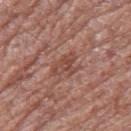Assessment: Part of a total-body skin-imaging series; this lesion was reviewed on a skin check and was not flagged for biopsy. Acquisition and patient details: A male patient, in their 80s. The lesion-visualizer software estimated a lesion area of about 5.5 mm² and an eccentricity of roughly 0.75. And it measured an average lesion color of about L≈47 a*≈23 b*≈26 (CIELAB), roughly 8 lightness units darker than nearby skin, and a normalized border contrast of about 6. The lesion is on the right thigh. The recorded lesion diameter is about 3.5 mm. Imaged with white-light lighting. A region of skin cropped from a whole-body photographic capture, roughly 15 mm wide.